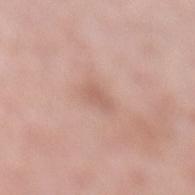No biopsy was performed on this lesion — it was imaged during a full skin examination and was not determined to be concerning. The subject is a female aged approximately 50. A 15 mm close-up extracted from a 3D total-body photography capture. The tile uses white-light illumination. The lesion is located on the left lower leg.